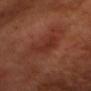Captured during whole-body skin photography for melanoma surveillance; the lesion was not biopsied. Cropped from a total-body skin-imaging series; the visible field is about 15 mm. The lesion-visualizer software estimated a peripheral color-asymmetry measure near 1. The analysis additionally found a classifier nevus-likeness of about 0/100 and lesion-presence confidence of about 85/100. The patient is a male roughly 50 years of age. Located on the head or neck. The tile uses cross-polarized illumination.